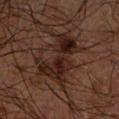* biopsy status — no biopsy performed (imaged during a skin exam)
* site — the right forearm
* acquisition — ~15 mm tile from a whole-body skin photo
* patient — male, in their 70s
* lighting — cross-polarized
* lesion diameter — ~7 mm (longest diameter)